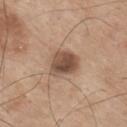• follow-up — no biopsy performed (imaged during a skin exam)
• subject — male, aged around 75
• automated metrics — a footprint of about 11 mm², a shape eccentricity near 0.65, and two-axis asymmetry of about 0.2; a mean CIELAB color near L≈51 a*≈17 b*≈28 and a lesion-to-skin contrast of about 9.5 (normalized; higher = more distinct); a detector confidence of about 100 out of 100 that the crop contains a lesion
• site — the mid back
• image source — ~15 mm tile from a whole-body skin photo